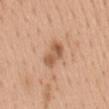follow-up = no biopsy performed (imaged during a skin exam) | illumination = white-light illumination | TBP lesion metrics = a lesion color around L≈57 a*≈22 b*≈34 in CIELAB and roughly 11 lightness units darker than nearby skin; a lesion-detection confidence of about 100/100 | image source = 15 mm crop, total-body photography | lesion size = about 3.5 mm | body site = the mid back | patient = female, aged 48 to 52.